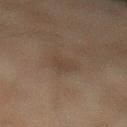Findings:
• notes — imaged on a skin check; not biopsied
• acquisition — ~15 mm tile from a whole-body skin photo
• anatomic site — the left lower leg
• subject — male, aged around 45
• image-analysis metrics — a border-irregularity index near 3.5/10, a within-lesion color-variation index near 1.5/10, and radial color variation of about 0.5
• illumination — cross-polarized